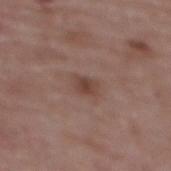Q: Is there a histopathology result?
A: total-body-photography surveillance lesion; no biopsy
Q: What kind of image is this?
A: ~15 mm tile from a whole-body skin photo
Q: How large is the lesion?
A: about 2.5 mm
Q: What are the patient's age and sex?
A: female, aged 63–67
Q: How was the tile lit?
A: white-light illumination
Q: What is the anatomic site?
A: the upper back